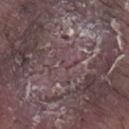| field | value |
|---|---|
| lesion size | ~1.5 mm (longest diameter) |
| TBP lesion metrics | a footprint of about 1 mm², an eccentricity of roughly 0.85, and a shape-asymmetry score of about 0.5 (0 = symmetric); a mean CIELAB color near L≈40 a*≈18 b*≈13, about 4 CIELAB-L* units darker than the surrounding skin, and a lesion-to-skin contrast of about 3.5 (normalized; higher = more distinct); border irregularity of about 4.5 on a 0–10 scale, a color-variation rating of about 0/10, and a peripheral color-asymmetry measure near 0 |
| patient | male, in their mid- to late 70s |
| anatomic site | the right lower leg |
| imaging modality | ~15 mm crop, total-body skin-cancer survey |
| pathology | an invasive squamous cell carcinoma (malignant) |